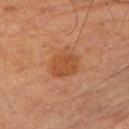Assessment: The lesion was photographed on a routine skin check and not biopsied; there is no pathology result. Background: The tile uses cross-polarized illumination. A 15 mm close-up tile from a total-body photography series done for melanoma screening. The total-body-photography lesion software estimated a lesion area of about 8.5 mm², an outline eccentricity of about 0.55 (0 = round, 1 = elongated), and a shape-asymmetry score of about 0.2 (0 = symmetric). The software also gave an average lesion color of about L≈40 a*≈22 b*≈32 (CIELAB) and a normalized lesion–skin contrast near 6.5. A male subject in their 70s. The recorded lesion diameter is about 4 mm. From the left upper arm.